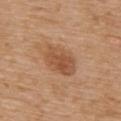notes: imaged on a skin check; not biopsied | tile lighting: white-light | subject: male, in their 70s | acquisition: 15 mm crop, total-body photography | location: the back.